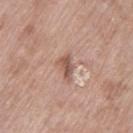Imaged with white-light lighting.
The subject is a female about 75 years old.
Measured at roughly 3 mm in maximum diameter.
A region of skin cropped from a whole-body photographic capture, roughly 15 mm wide.
From the left thigh.
The total-body-photography lesion software estimated a border-irregularity index near 4/10 and peripheral color asymmetry of about 1.5.